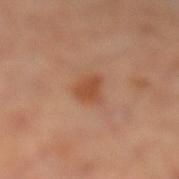Acquisition and patient details:
A close-up tile cropped from a whole-body skin photograph, about 15 mm across. The lesion is on the left lower leg. Captured under cross-polarized illumination. A female patient, approximately 60 years of age.